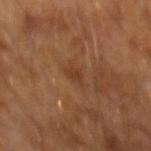{"biopsy_status": "not biopsied; imaged during a skin examination", "image": {"source": "total-body photography crop", "field_of_view_mm": 15}, "automated_metrics": {"border_irregularity_0_10": 3.5, "color_variation_0_10": 1.5, "peripheral_color_asymmetry": 0.5, "nevus_likeness_0_100": 0}, "patient": {"sex": "male", "age_approx": 65}, "lesion_size": {"long_diameter_mm_approx": 2.5}, "lighting": "cross-polarized"}